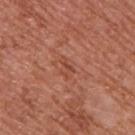From the back.
A male patient aged 53–57.
A 15 mm crop from a total-body photograph taken for skin-cancer surveillance.
Automated image analysis of the tile measured a footprint of about 2.5 mm², a shape eccentricity near 0.9, and two-axis asymmetry of about 0.45.